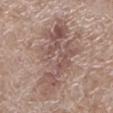The lesion was photographed on a routine skin check and not biopsied; there is no pathology result.
On the left lower leg.
This is a white-light tile.
A 15 mm crop from a total-body photograph taken for skin-cancer surveillance.
Approximately 10.5 mm at its widest.
A male subject roughly 70 years of age.